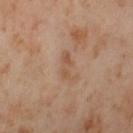follow-up = catalogued during a skin exam; not biopsied
body site = the left thigh
image source = ~15 mm tile from a whole-body skin photo
patient = female, aged 53 to 57
lighting = cross-polarized
TBP lesion metrics = a footprint of about 4 mm², an eccentricity of roughly 0.9, and two-axis asymmetry of about 0.4; a lesion color around L≈54 a*≈19 b*≈32 in CIELAB, roughly 7 lightness units darker than nearby skin, and a normalized border contrast of about 5.5; a border-irregularity index near 5/10, a within-lesion color-variation index near 0/10, and peripheral color asymmetry of about 0
diameter = about 3.5 mm